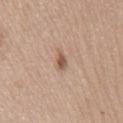  biopsy_status: not biopsied; imaged during a skin examination
  patient:
    sex: female
    age_approx: 65
  lighting: white-light
  lesion_size:
    long_diameter_mm_approx: 3.0
  site: chest
  image:
    source: total-body photography crop
    field_of_view_mm: 15